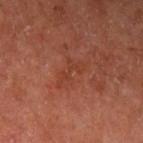* workup — total-body-photography surveillance lesion; no biopsy
* automated metrics — roughly 5 lightness units darker than nearby skin and a lesion-to-skin contrast of about 4.5 (normalized; higher = more distinct); border irregularity of about 9 on a 0–10 scale and a color-variation rating of about 0/10; a classifier nevus-likeness of about 0/100 and lesion-presence confidence of about 100/100
* lighting — cross-polarized illumination
* image source — ~15 mm tile from a whole-body skin photo
* patient — male, roughly 65 years of age
* body site — the left upper arm